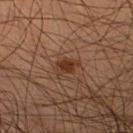No biopsy was performed on this lesion — it was imaged during a full skin examination and was not determined to be concerning.